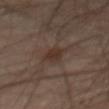Part of a total-body skin-imaging series; this lesion was reviewed on a skin check and was not flagged for biopsy.
From the abdomen.
A close-up tile cropped from a whole-body skin photograph, about 15 mm across.
The tile uses cross-polarized illumination.
A male subject, in their mid-60s.
Automated tile analysis of the lesion measured a footprint of about 6 mm², a shape eccentricity near 0.8, and a symmetry-axis asymmetry near 0.3.
About 3.5 mm across.